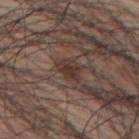Captured during whole-body skin photography for melanoma surveillance; the lesion was not biopsied. A male subject, in their 70s. A roughly 15 mm field-of-view crop from a total-body skin photograph. Measured at roughly 3 mm in maximum diameter. On the upper back. The lesion-visualizer software estimated a lesion color around L≈35 a*≈16 b*≈20 in CIELAB, roughly 9 lightness units darker than nearby skin, and a normalized lesion–skin contrast near 8. The software also gave border irregularity of about 2.5 on a 0–10 scale and internal color variation of about 2 on a 0–10 scale.